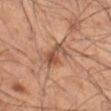No biopsy was performed on this lesion — it was imaged during a full skin examination and was not determined to be concerning.
The lesion is located on the left thigh.
A 15 mm crop from a total-body photograph taken for skin-cancer surveillance.
Imaged with cross-polarized lighting.
Longest diameter approximately 3.5 mm.
The patient is a male approximately 55 years of age.